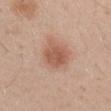A lesion tile, about 15 mm wide, cut from a 3D total-body photograph. The patient is a male aged 28 to 32. Measured at roughly 4 mm in maximum diameter. Imaged with white-light lighting. Located on the right upper arm.